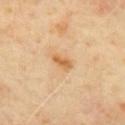Q: Was a biopsy performed?
A: imaged on a skin check; not biopsied
Q: What is the anatomic site?
A: the back
Q: What lighting was used for the tile?
A: cross-polarized
Q: Lesion size?
A: ~3 mm (longest diameter)
Q: What kind of image is this?
A: total-body-photography crop, ~15 mm field of view
Q: Who is the patient?
A: male, aged 63 to 67
Q: What did automated image analysis measure?
A: an outline eccentricity of about 0.85 (0 = round, 1 = elongated) and two-axis asymmetry of about 0.25; a border-irregularity index near 2.5/10 and a within-lesion color-variation index near 2/10